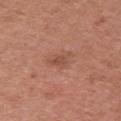| field | value |
|---|---|
| follow-up | imaged on a skin check; not biopsied |
| acquisition | total-body-photography crop, ~15 mm field of view |
| subject | female, aged 48–52 |
| diameter | about 2.5 mm |
| illumination | white-light illumination |
| automated lesion analysis | a nevus-likeness score of about 5/100 and lesion-presence confidence of about 100/100 |
| body site | the arm |